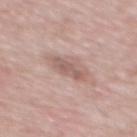Q: Was this lesion biopsied?
A: catalogued during a skin exam; not biopsied
Q: Lesion size?
A: ≈4 mm
Q: What did automated image analysis measure?
A: a mean CIELAB color near L≈58 a*≈19 b*≈23, a lesion–skin lightness drop of about 10, and a normalized border contrast of about 6.5; a classifier nevus-likeness of about 10/100 and lesion-presence confidence of about 100/100
Q: What are the patient's age and sex?
A: male, in their 50s
Q: Where on the body is the lesion?
A: the mid back
Q: How was this image acquired?
A: ~15 mm crop, total-body skin-cancer survey